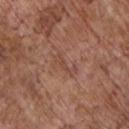workup = catalogued during a skin exam; not biopsied
acquisition = total-body-photography crop, ~15 mm field of view
diameter = ≈3 mm
anatomic site = the chest
illumination = white-light illumination
patient = male, in their 70s